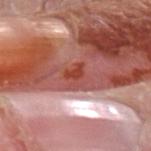The lesion is located on the left forearm. Automated image analysis of the tile measured an area of roughly 5 mm², an outline eccentricity of about 0.6 (0 = round, 1 = elongated), and a symmetry-axis asymmetry near 0.25. The software also gave a border-irregularity rating of about 3/10, internal color variation of about 5.5 on a 0–10 scale, and a peripheral color-asymmetry measure near 1.5. And it measured an automated nevus-likeness rating near 0 out of 100. A male subject, about 40 years old. This image is a 15 mm lesion crop taken from a total-body photograph. Longest diameter approximately 3 mm. Imaged with white-light lighting.